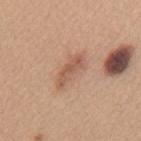{"automated_metrics": {"color_variation_0_10": 1.5, "peripheral_color_asymmetry": 0.5, "nevus_likeness_0_100": 50}, "site": "upper back", "patient": {"sex": "female", "age_approx": 40}, "lighting": "white-light", "image": {"source": "total-body photography crop", "field_of_view_mm": 15}}